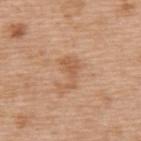notes=total-body-photography surveillance lesion; no biopsy
site=the upper back
image=15 mm crop, total-body photography
size=~3.5 mm (longest diameter)
illumination=white-light illumination
patient=female, roughly 55 years of age